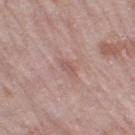  biopsy_status: not biopsied; imaged during a skin examination
  site: right thigh
  image:
    source: total-body photography crop
    field_of_view_mm: 15
  patient:
    sex: female
    age_approx: 70